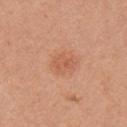Q: Was a biopsy performed?
A: imaged on a skin check; not biopsied
Q: How large is the lesion?
A: ~2.5 mm (longest diameter)
Q: Where on the body is the lesion?
A: the right upper arm
Q: What did automated image analysis measure?
A: a classifier nevus-likeness of about 40/100 and a detector confidence of about 100 out of 100 that the crop contains a lesion
Q: What kind of image is this?
A: 15 mm crop, total-body photography
Q: Who is the patient?
A: female, approximately 40 years of age
Q: How was the tile lit?
A: white-light illumination